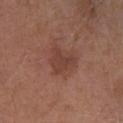A male subject aged around 65.
From the right lower leg.
An algorithmic analysis of the crop reported an area of roughly 5.5 mm² and an eccentricity of roughly 0.4. The software also gave a lesion–skin lightness drop of about 7 and a normalized lesion–skin contrast near 6.
A region of skin cropped from a whole-body photographic capture, roughly 15 mm wide.
Measured at roughly 3 mm in maximum diameter.
The tile uses white-light illumination.